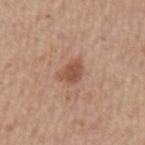notes: imaged on a skin check; not biopsied
diameter: ~3 mm (longest diameter)
tile lighting: white-light illumination
imaging modality: ~15 mm crop, total-body skin-cancer survey
patient: male, aged 73 to 77
body site: the left upper arm